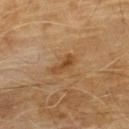follow-up: catalogued during a skin exam; not biopsied | patient: male, roughly 60 years of age | acquisition: ~15 mm tile from a whole-body skin photo | location: the chest.